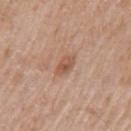workup: no biopsy performed (imaged during a skin exam) | size: ~3 mm (longest diameter) | site: the left upper arm | subject: male, aged 63–67 | imaging modality: 15 mm crop, total-body photography | illumination: white-light | TBP lesion metrics: an outline eccentricity of about 0.8 (0 = round, 1 = elongated) and two-axis asymmetry of about 0.25; a lesion color around L≈55 a*≈21 b*≈31 in CIELAB, a lesion–skin lightness drop of about 9, and a normalized lesion–skin contrast near 7; a border-irregularity rating of about 2/10, internal color variation of about 3.5 on a 0–10 scale, and peripheral color asymmetry of about 1.5; an automated nevus-likeness rating near 25 out of 100 and lesion-presence confidence of about 100/100.